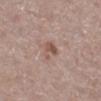| feature | finding |
|---|---|
| follow-up | no biopsy performed (imaged during a skin exam) |
| site | the right lower leg |
| patient | female, in their 50s |
| imaging modality | 15 mm crop, total-body photography |